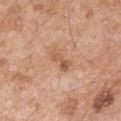tile lighting — white-light
image — 15 mm crop, total-body photography
TBP lesion metrics — border irregularity of about 4.5 on a 0–10 scale, a within-lesion color-variation index near 4/10, and radial color variation of about 1; a classifier nevus-likeness of about 0/100 and lesion-presence confidence of about 100/100
patient — male, aged 53 to 57
location — the right upper arm